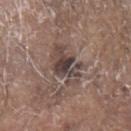biopsy status: no biopsy performed (imaged during a skin exam)
patient: male, roughly 80 years of age
illumination: white-light
imaging modality: ~15 mm tile from a whole-body skin photo
lesion size: ~4.5 mm (longest diameter)
anatomic site: the head or neck